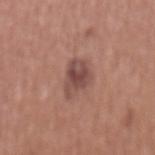The lesion was photographed on a routine skin check and not biopsied; there is no pathology result. On the abdomen. A male patient, aged 43 to 47. A lesion tile, about 15 mm wide, cut from a 3D total-body photograph.